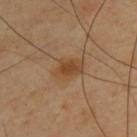biopsy status — imaged on a skin check; not biopsied | image — ~15 mm tile from a whole-body skin photo | diameter — ≈4 mm | site — the back | lighting — cross-polarized illumination | automated metrics — a border-irregularity index near 2/10, a color-variation rating of about 3/10, and radial color variation of about 1 | patient — male, approximately 65 years of age.